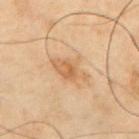Case summary:
* biopsy status · total-body-photography surveillance lesion; no biopsy
* body site · the chest
* lesion diameter · about 4.5 mm
* lighting · cross-polarized illumination
* patient · male, aged 53–57
* imaging modality · ~15 mm tile from a whole-body skin photo
* automated lesion analysis · a lesion–skin lightness drop of about 8 and a normalized border contrast of about 6; a border-irregularity rating of about 3/10, internal color variation of about 3 on a 0–10 scale, and peripheral color asymmetry of about 1